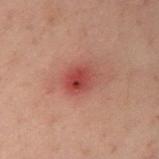Recorded during total-body skin imaging; not selected for excision or biopsy.